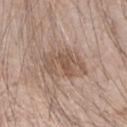A roughly 15 mm field-of-view crop from a total-body skin photograph. The total-body-photography lesion software estimated a nevus-likeness score of about 0/100. Longest diameter approximately 5.5 mm. Captured under white-light illumination. From the right upper arm. The patient is a male aged 23 to 27.